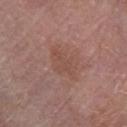Imaged during a routine full-body skin examination; the lesion was not biopsied and no histopathology is available.
A roughly 15 mm field-of-view crop from a total-body skin photograph.
From the left lower leg.
Longest diameter approximately 4.5 mm.
Automated image analysis of the tile measured a lesion color around L≈49 a*≈21 b*≈25 in CIELAB and a normalized lesion–skin contrast near 4.5. The analysis additionally found a detector confidence of about 100 out of 100 that the crop contains a lesion.
The subject is a male aged 78–82.
The tile uses white-light illumination.